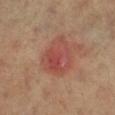automated metrics: a border-irregularity index near 3/10, internal color variation of about 5 on a 0–10 scale, and peripheral color asymmetry of about 1.5; acquisition: 15 mm crop, total-body photography; patient: female, roughly 65 years of age; body site: the leg; lesion diameter: ~4.5 mm (longest diameter).